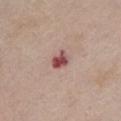• biopsy status: catalogued during a skin exam; not biopsied
• patient: female, in their mid-60s
• imaging modality: ~15 mm crop, total-body skin-cancer survey
• body site: the chest
• illumination: white-light illumination
• diameter: about 2.5 mm